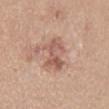Captured during whole-body skin photography for melanoma surveillance; the lesion was not biopsied. Measured at roughly 4.5 mm in maximum diameter. Located on the mid back. A female subject, approximately 40 years of age. Cropped from a total-body skin-imaging series; the visible field is about 15 mm.